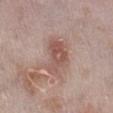<record>
<biopsy_status>not biopsied; imaged during a skin examination</biopsy_status>
<lesion_size>
  <long_diameter_mm_approx>4.5</long_diameter_mm_approx>
</lesion_size>
<image>
  <source>total-body photography crop</source>
  <field_of_view_mm>15</field_of_view_mm>
</image>
<site>right lower leg</site>
<patient>
  <sex>male</sex>
  <age_approx>60</age_approx>
</patient>
</record>